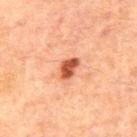No biopsy was performed on this lesion — it was imaged during a full skin examination and was not determined to be concerning. This is a cross-polarized tile. A roughly 15 mm field-of-view crop from a total-body skin photograph. A male subject, aged 58–62. Automated tile analysis of the lesion measured a mean CIELAB color near L≈42 a*≈25 b*≈30, roughly 14 lightness units darker than nearby skin, and a lesion-to-skin contrast of about 10.5 (normalized; higher = more distinct). Located on the upper back.